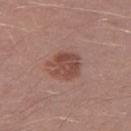biopsy status=no biopsy performed (imaged during a skin exam)
lighting=white-light illumination
anatomic site=the leg
diameter=~4 mm (longest diameter)
subject=male, in their 30s
imaging modality=15 mm crop, total-body photography